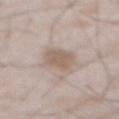notes: total-body-photography surveillance lesion; no biopsy
patient: male, aged approximately 50
acquisition: total-body-photography crop, ~15 mm field of view
anatomic site: the abdomen